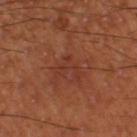follow-up: no biopsy performed (imaged during a skin exam) | diameter: ~3.5 mm (longest diameter) | automated lesion analysis: a lesion area of about 4.5 mm², a shape eccentricity near 0.65, and a shape-asymmetry score of about 0.7 (0 = symmetric); an average lesion color of about L≈36 a*≈27 b*≈30 (CIELAB) and roughly 5 lightness units darker than nearby skin; a detector confidence of about 100 out of 100 that the crop contains a lesion | subject: male, in their mid-50s | illumination: cross-polarized illumination | image source: 15 mm crop, total-body photography | body site: the left thigh.